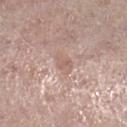A close-up tile cropped from a whole-body skin photograph, about 15 mm across.
Captured under white-light illumination.
About 3 mm across.
On the right lower leg.
The subject is a female aged 63 to 67.